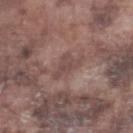biopsy status = catalogued during a skin exam; not biopsied
size = about 3.5 mm
image = ~15 mm crop, total-body skin-cancer survey
subject = male, aged around 75
site = the right lower leg
tile lighting = white-light